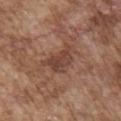Captured during whole-body skin photography for melanoma surveillance; the lesion was not biopsied. The lesion is on the chest. A close-up tile cropped from a whole-body skin photograph, about 15 mm across. About 4.5 mm across. This is a white-light tile. A male subject aged 73 to 77.